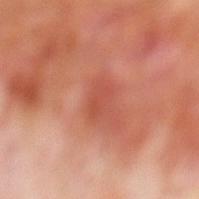The lesion was tiled from a total-body skin photograph and was not biopsied.